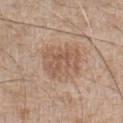Q: Was a biopsy performed?
A: no biopsy performed (imaged during a skin exam)
Q: What is the anatomic site?
A: the abdomen
Q: What are the patient's age and sex?
A: male, aged approximately 70
Q: Lesion size?
A: about 4.5 mm
Q: What is the imaging modality?
A: ~15 mm crop, total-body skin-cancer survey
Q: What lighting was used for the tile?
A: white-light illumination